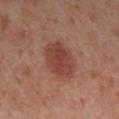notes — catalogued during a skin exam; not biopsied
patient — female, about 55 years old
imaging modality — ~15 mm crop, total-body skin-cancer survey
automated lesion analysis — a border-irregularity index near 1.5/10 and a color-variation rating of about 3.5/10
body site — the right lower leg
tile lighting — cross-polarized